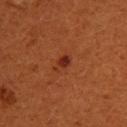Notes:
* workup · total-body-photography surveillance lesion; no biopsy
* illumination · cross-polarized illumination
* patient · female, aged 28 to 32
* image · 15 mm crop, total-body photography
* site · the upper back
* diameter · about 2.5 mm